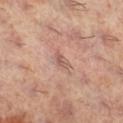Impression: Recorded during total-body skin imaging; not selected for excision or biopsy. Background: This is a cross-polarized tile. A lesion tile, about 15 mm wide, cut from a 3D total-body photograph. A female subject, in their 60s. The lesion is on the right lower leg. About 2.5 mm across.